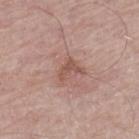biopsy_status: not biopsied; imaged during a skin examination
lesion_size:
  long_diameter_mm_approx: 3.0
image:
  source: total-body photography crop
  field_of_view_mm: 15
site: left thigh
lighting: white-light
automated_metrics:
  cielab_L: 53
  cielab_a: 21
  cielab_b: 26
  vs_skin_darker_L: 9.0
  vs_skin_contrast_norm: 6.5
  border_irregularity_0_10: 7.0
  color_variation_0_10: 0.5
  peripheral_color_asymmetry: 0.0
  nevus_likeness_0_100: 0
  lesion_detection_confidence_0_100: 100
patient:
  sex: male
  age_approx: 75